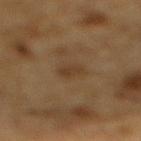Impression:
Part of a total-body skin-imaging series; this lesion was reviewed on a skin check and was not flagged for biopsy.
Background:
The recorded lesion diameter is about 2.5 mm. A male patient aged approximately 85 or older. The lesion is located on the mid back. Imaged with cross-polarized lighting. This image is a 15 mm lesion crop taken from a total-body photograph. The lesion-visualizer software estimated a lesion area of about 3 mm² and two-axis asymmetry of about 0.3. The analysis additionally found a mean CIELAB color near L≈32 a*≈13 b*≈27, about 5 CIELAB-L* units darker than the surrounding skin, and a normalized lesion–skin contrast near 5.5. The analysis additionally found border irregularity of about 3 on a 0–10 scale and peripheral color asymmetry of about 0.